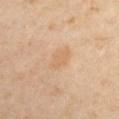Clinical summary: Captured under cross-polarized illumination. A lesion tile, about 15 mm wide, cut from a 3D total-body photograph. From the right upper arm. Measured at roughly 3.5 mm in maximum diameter. A male subject roughly 40 years of age. An algorithmic analysis of the crop reported a footprint of about 5 mm², an eccentricity of roughly 0.8, and two-axis asymmetry of about 0.2.